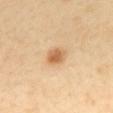Q: Was a biopsy performed?
A: no biopsy performed (imaged during a skin exam)
Q: How large is the lesion?
A: ≈2.5 mm
Q: What are the patient's age and sex?
A: female, aged approximately 40
Q: What did automated image analysis measure?
A: a mean CIELAB color near L≈65 a*≈21 b*≈41, roughly 12 lightness units darker than nearby skin, and a normalized border contrast of about 8
Q: What kind of image is this?
A: 15 mm crop, total-body photography
Q: Illumination type?
A: cross-polarized illumination
Q: Lesion location?
A: the mid back